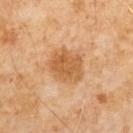{
  "biopsy_status": "not biopsied; imaged during a skin examination",
  "lighting": "cross-polarized",
  "automated_metrics": {
    "cielab_L": 57,
    "cielab_a": 22,
    "cielab_b": 40,
    "vs_skin_darker_L": 10.0,
    "vs_skin_contrast_norm": 7.5,
    "border_irregularity_0_10": 2.0,
    "color_variation_0_10": 3.5,
    "peripheral_color_asymmetry": 1.5,
    "nevus_likeness_0_100": 30,
    "lesion_detection_confidence_0_100": 100
  },
  "image": {
    "source": "total-body photography crop",
    "field_of_view_mm": 15
  },
  "patient": {
    "sex": "male",
    "age_approx": 65
  },
  "lesion_size": {
    "long_diameter_mm_approx": 4.5
  }
}